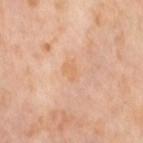Assessment: This lesion was catalogued during total-body skin photography and was not selected for biopsy. Acquisition and patient details: Automated image analysis of the tile measured an area of roughly 3.5 mm², a shape eccentricity near 0.75, and two-axis asymmetry of about 0.3. The analysis additionally found a normalized lesion–skin contrast near 4.5. The analysis additionally found border irregularity of about 2.5 on a 0–10 scale, a color-variation rating of about 1/10, and radial color variation of about 0.5. The software also gave a lesion-detection confidence of about 100/100. On the right thigh. A lesion tile, about 15 mm wide, cut from a 3D total-body photograph. A female patient, about 55 years old. The recorded lesion diameter is about 2.5 mm.